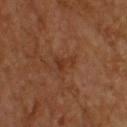workup = imaged on a skin check; not biopsied | TBP lesion metrics = internal color variation of about 0.5 on a 0–10 scale and peripheral color asymmetry of about 0.5; an automated nevus-likeness rating near 0 out of 100 and lesion-presence confidence of about 100/100 | image = ~15 mm crop, total-body skin-cancer survey | anatomic site = the upper back | patient = male, about 60 years old | lesion size = about 3 mm | tile lighting = cross-polarized illumination.